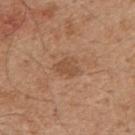Clinical impression: The lesion was photographed on a routine skin check and not biopsied; there is no pathology result. Image and clinical context: The recorded lesion diameter is about 3 mm. A male patient, about 45 years old. The lesion-visualizer software estimated a mean CIELAB color near L≈50 a*≈21 b*≈33 and a lesion–skin lightness drop of about 8. It also reported border irregularity of about 3 on a 0–10 scale, a color-variation rating of about 1/10, and a peripheral color-asymmetry measure near 0.5. The lesion is located on the mid back. The tile uses white-light illumination. Cropped from a whole-body photographic skin survey; the tile spans about 15 mm.